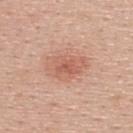Q: Is there a histopathology result?
A: no biopsy performed (imaged during a skin exam)
Q: Lesion size?
A: ≈5 mm
Q: What is the anatomic site?
A: the upper back
Q: What is the imaging modality?
A: 15 mm crop, total-body photography
Q: Who is the patient?
A: female, roughly 40 years of age
Q: How was the tile lit?
A: white-light